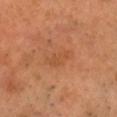Impression: Imaged during a routine full-body skin examination; the lesion was not biopsied and no histopathology is available. Image and clinical context: Located on the head or neck. A male subject in their 50s. Measured at roughly 4.5 mm in maximum diameter. Imaged with cross-polarized lighting. A lesion tile, about 15 mm wide, cut from a 3D total-body photograph.